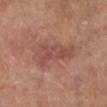Part of a total-body skin-imaging series; this lesion was reviewed on a skin check and was not flagged for biopsy. Captured under cross-polarized illumination. A 15 mm close-up extracted from a 3D total-body photography capture. A female patient aged 73–77. Located on the left lower leg.